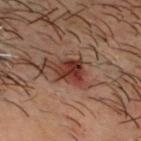Recorded during total-body skin imaging; not selected for excision or biopsy.
The subject is a male aged approximately 50.
Imaged with cross-polarized lighting.
A region of skin cropped from a whole-body photographic capture, roughly 15 mm wide.
The lesion is on the head or neck.